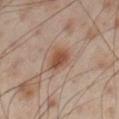No biopsy was performed on this lesion — it was imaged during a full skin examination and was not determined to be concerning. A 15 mm close-up extracted from a 3D total-body photography capture. Approximately 3 mm at its widest. On the right thigh. The tile uses cross-polarized illumination. A male patient in their mid- to late 50s.